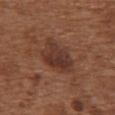| key | value |
|---|---|
| follow-up | total-body-photography surveillance lesion; no biopsy |
| site | the chest |
| lesion size | about 5 mm |
| subject | female, aged around 75 |
| image | 15 mm crop, total-body photography |
| lighting | white-light |
| image-analysis metrics | an area of roughly 13 mm², an outline eccentricity of about 0.8 (0 = round, 1 = elongated), and a shape-asymmetry score of about 0.25 (0 = symmetric); roughly 9 lightness units darker than nearby skin and a lesion-to-skin contrast of about 8 (normalized; higher = more distinct); an automated nevus-likeness rating near 35 out of 100 and a lesion-detection confidence of about 100/100 |